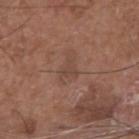Recorded during total-body skin imaging; not selected for excision or biopsy. The lesion is on the head or neck. A 15 mm close-up tile from a total-body photography series done for melanoma screening. A male subject aged 68–72. The lesion's longest dimension is about 2.5 mm. This is a white-light tile.